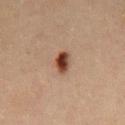Impression: Part of a total-body skin-imaging series; this lesion was reviewed on a skin check and was not flagged for biopsy. Acquisition and patient details: A male patient, in their mid- to late 40s. Cropped from a total-body skin-imaging series; the visible field is about 15 mm. Located on the front of the torso. Measured at roughly 3 mm in maximum diameter. Automated image analysis of the tile measured an area of roughly 4.5 mm², an outline eccentricity of about 0.75 (0 = round, 1 = elongated), and a shape-asymmetry score of about 0.15 (0 = symmetric). And it measured a mean CIELAB color near L≈35 a*≈19 b*≈25, roughly 14 lightness units darker than nearby skin, and a lesion-to-skin contrast of about 12 (normalized; higher = more distinct). The analysis additionally found lesion-presence confidence of about 100/100.